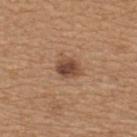Captured during whole-body skin photography for melanoma surveillance; the lesion was not biopsied. The lesion is on the upper back. An algorithmic analysis of the crop reported a footprint of about 6 mm², an eccentricity of roughly 0.65, and two-axis asymmetry of about 0.15. The analysis additionally found border irregularity of about 1.5 on a 0–10 scale, a within-lesion color-variation index near 4.5/10, and peripheral color asymmetry of about 1.5. The analysis additionally found a nevus-likeness score of about 90/100. The patient is a female roughly 45 years of age. A close-up tile cropped from a whole-body skin photograph, about 15 mm across. Approximately 3 mm at its widest.